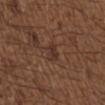  biopsy_status: not biopsied; imaged during a skin examination
  site: upper back
  automated_metrics:
    nevus_likeness_0_100: 0
    lesion_detection_confidence_0_100: 95
  patient:
    sex: male
    age_approx: 50
  lesion_size:
    long_diameter_mm_approx: 2.5
  lighting: white-light
  image:
    source: total-body photography crop
    field_of_view_mm: 15